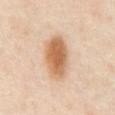notes=no biopsy performed (imaged during a skin exam)
patient=male, aged around 65
site=the front of the torso
TBP lesion metrics=an area of roughly 15 mm² and two-axis asymmetry of about 0.2; a classifier nevus-likeness of about 100/100
lighting=cross-polarized illumination
acquisition=~15 mm tile from a whole-body skin photo